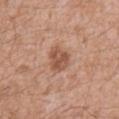Recorded during total-body skin imaging; not selected for excision or biopsy.
Approximately 3.5 mm at its widest.
The lesion is on the mid back.
The patient is a male aged approximately 60.
A 15 mm crop from a total-body photograph taken for skin-cancer surveillance.